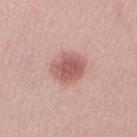Notes:
- follow-up — imaged on a skin check; not biopsied
- imaging modality — total-body-photography crop, ~15 mm field of view
- TBP lesion metrics — a border-irregularity index near 2/10, a within-lesion color-variation index near 3/10, and a peripheral color-asymmetry measure near 1; a detector confidence of about 100 out of 100 that the crop contains a lesion
- tile lighting — white-light illumination
- lesion size — about 4 mm
- subject — female, about 25 years old
- location — the left thigh